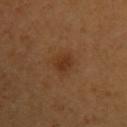Case summary:
– notes: total-body-photography surveillance lesion; no biopsy
– tile lighting: cross-polarized illumination
– body site: the left upper arm
– subject: female, roughly 45 years of age
– automated metrics: a mean CIELAB color near L≈33 a*≈20 b*≈32, roughly 7 lightness units darker than nearby skin, and a normalized lesion–skin contrast near 6.5; internal color variation of about 2 on a 0–10 scale and peripheral color asymmetry of about 0.5; a nevus-likeness score of about 90/100 and lesion-presence confidence of about 100/100
– imaging modality: total-body-photography crop, ~15 mm field of view
– size: about 3 mm